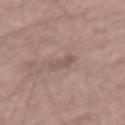A male patient, roughly 60 years of age.
A lesion tile, about 15 mm wide, cut from a 3D total-body photograph.
Located on the mid back.
The lesion-visualizer software estimated an average lesion color of about L≈53 a*≈17 b*≈22 (CIELAB), a lesion–skin lightness drop of about 7, and a normalized border contrast of about 5. It also reported a border-irregularity rating of about 4/10.
This is a white-light tile.